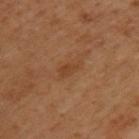Captured during whole-body skin photography for melanoma surveillance; the lesion was not biopsied. Automated image analysis of the tile measured a footprint of about 3.5 mm² and an outline eccentricity of about 0.85 (0 = round, 1 = elongated). It also reported a mean CIELAB color near L≈41 a*≈22 b*≈34. It also reported a border-irregularity index near 4/10, internal color variation of about 1.5 on a 0–10 scale, and peripheral color asymmetry of about 0.5. Imaged with cross-polarized lighting. On the upper back. The subject is a male aged approximately 50. The recorded lesion diameter is about 3 mm. Cropped from a total-body skin-imaging series; the visible field is about 15 mm.